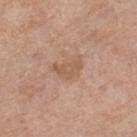* workup · total-body-photography surveillance lesion; no biopsy
* lesion diameter · ≈4 mm
* body site · the right lower leg
* image · 15 mm crop, total-body photography
* patient · female, aged approximately 70